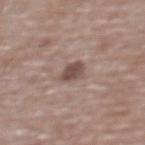location: the upper back; patient: female, in their mid- to late 60s; image source: ~15 mm tile from a whole-body skin photo.